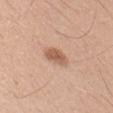Case summary:
- biopsy status — imaged on a skin check; not biopsied
- acquisition — 15 mm crop, total-body photography
- automated metrics — a shape eccentricity near 0.75 and a symmetry-axis asymmetry near 0.2; a classifier nevus-likeness of about 95/100
- size — ≈3 mm
- illumination — white-light illumination
- subject — male, aged around 20
- location — the right upper arm